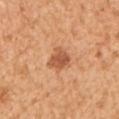Captured during whole-body skin photography for melanoma surveillance; the lesion was not biopsied.
Located on the left upper arm.
The recorded lesion diameter is about 3 mm.
Captured under white-light illumination.
Cropped from a whole-body photographic skin survey; the tile spans about 15 mm.
An algorithmic analysis of the crop reported an area of roughly 6.5 mm² and a shape eccentricity near 0.5. The software also gave a border-irregularity index near 2.5/10, internal color variation of about 3.5 on a 0–10 scale, and peripheral color asymmetry of about 1.
A male subject, roughly 55 years of age.